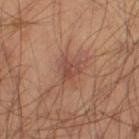image source = 15 mm crop, total-body photography | automated lesion analysis = an area of roughly 4.5 mm² and a shape eccentricity near 0.3 | anatomic site = the leg | subject = male, aged 38 to 42 | illumination = cross-polarized | size = ≈3 mm.